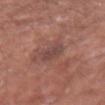Impression:
This lesion was catalogued during total-body skin photography and was not selected for biopsy.
Context:
This is a white-light tile. From the right forearm. Cropped from a whole-body photographic skin survey; the tile spans about 15 mm. Longest diameter approximately 3 mm. The patient is a male roughly 65 years of age. An algorithmic analysis of the crop reported an area of roughly 5.5 mm², an outline eccentricity of about 0.5 (0 = round, 1 = elongated), and a shape-asymmetry score of about 0.2 (0 = symmetric). And it measured a mean CIELAB color near L≈44 a*≈21 b*≈22, roughly 7 lightness units darker than nearby skin, and a normalized border contrast of about 6. The analysis additionally found border irregularity of about 2.5 on a 0–10 scale, internal color variation of about 1.5 on a 0–10 scale, and radial color variation of about 0.5. The software also gave an automated nevus-likeness rating near 0 out of 100 and a lesion-detection confidence of about 95/100.